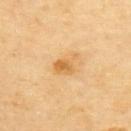Captured during whole-body skin photography for melanoma surveillance; the lesion was not biopsied.
The lesion is on the upper back.
An algorithmic analysis of the crop reported a footprint of about 5 mm² and a shape-asymmetry score of about 0.35 (0 = symmetric). The software also gave a lesion-detection confidence of about 100/100.
The patient is a female aged 68–72.
A 15 mm crop from a total-body photograph taken for skin-cancer surveillance.